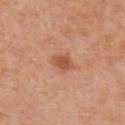{
  "biopsy_status": "not biopsied; imaged during a skin examination",
  "patient": {
    "sex": "male",
    "age_approx": 70
  },
  "automated_metrics": {
    "vs_skin_darker_L": 10.0,
    "vs_skin_contrast_norm": 7.0,
    "nevus_likeness_0_100": 75,
    "lesion_detection_confidence_0_100": 100
  },
  "image": {
    "source": "total-body photography crop",
    "field_of_view_mm": 15
  },
  "lighting": "white-light",
  "site": "front of the torso"
}